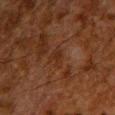| key | value |
|---|---|
| workup | catalogued during a skin exam; not biopsied |
| automated metrics | a lesion area of about 3.5 mm² and two-axis asymmetry of about 0.4; a lesion color around L≈22 a*≈17 b*≈24 in CIELAB, roughly 4 lightness units darker than nearby skin, and a normalized border contrast of about 5; a border-irregularity index near 4/10 and radial color variation of about 0.5 |
| body site | the chest |
| lighting | cross-polarized illumination |
| size | about 2.5 mm |
| image source | total-body-photography crop, ~15 mm field of view |
| subject | male, aged around 60 |